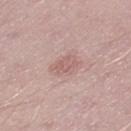  automated_metrics:
    area_mm2_approx: 5.5
    eccentricity: 0.7
    shape_asymmetry: 0.25
    cielab_L: 60
    cielab_a: 21
    cielab_b: 22
    vs_skin_contrast_norm: 5.0
    nevus_likeness_0_100: 0
  image:
    source: total-body photography crop
    field_of_view_mm: 15
  lesion_size:
    long_diameter_mm_approx: 3.0
  lighting: white-light
  site: right thigh
  patient:
    sex: male
    age_approx: 50